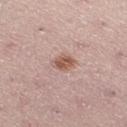Located on the left lower leg. A 15 mm close-up extracted from a 3D total-body photography capture. A female subject aged around 25.